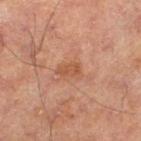About 3 mm across.
A 15 mm crop from a total-body photograph taken for skin-cancer surveillance.
The tile uses cross-polarized illumination.
The lesion is located on the left lower leg.
The patient is a male in their mid-60s.